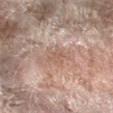Assessment: This lesion was catalogued during total-body skin photography and was not selected for biopsy. Acquisition and patient details: The total-body-photography lesion software estimated internal color variation of about 1.5 on a 0–10 scale and radial color variation of about 0.5. Cropped from a whole-body photographic skin survey; the tile spans about 15 mm. Captured under white-light illumination. The patient is a female aged approximately 75. On the right forearm. Longest diameter approximately 3 mm.